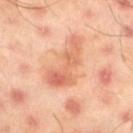Q: What are the patient's age and sex?
A: male, aged 43 to 47
Q: What is the anatomic site?
A: the left thigh
Q: What is the imaging modality?
A: ~15 mm tile from a whole-body skin photo